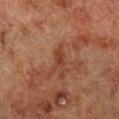Assessment:
The lesion was photographed on a routine skin check and not biopsied; there is no pathology result.
Image and clinical context:
A male subject, about 70 years old. The lesion is located on the right lower leg. The lesion's longest dimension is about 2.5 mm. Cropped from a whole-body photographic skin survey; the tile spans about 15 mm.